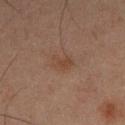Imaged during a routine full-body skin examination; the lesion was not biopsied and no histopathology is available.
Captured under cross-polarized illumination.
This image is a 15 mm lesion crop taken from a total-body photograph.
From the left lower leg.
A male subject, about 65 years old.
An algorithmic analysis of the crop reported an area of roughly 4.5 mm², an eccentricity of roughly 0.8, and a symmetry-axis asymmetry near 0.3. The software also gave a lesion color around L≈34 a*≈15 b*≈24 in CIELAB and a lesion-to-skin contrast of about 5.5 (normalized; higher = more distinct). The software also gave border irregularity of about 3 on a 0–10 scale, internal color variation of about 1.5 on a 0–10 scale, and a peripheral color-asymmetry measure near 0.5. The software also gave a detector confidence of about 100 out of 100 that the crop contains a lesion.
The lesion's longest dimension is about 3 mm.